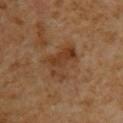On the left upper arm.
The patient is a male aged 58–62.
A 15 mm close-up extracted from a 3D total-body photography capture.